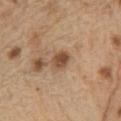Located on the left upper arm. A 15 mm close-up extracted from a 3D total-body photography capture. A male patient about 70 years old.